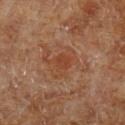Findings:
– biopsy status · imaged on a skin check; not biopsied
– patient · male, aged 68 to 72
– location · the left lower leg
– imaging modality · ~15 mm tile from a whole-body skin photo